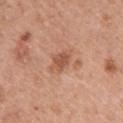| key | value |
|---|---|
| notes | catalogued during a skin exam; not biopsied |
| site | the left upper arm |
| imaging modality | total-body-photography crop, ~15 mm field of view |
| tile lighting | white-light illumination |
| subject | female, roughly 40 years of age |
| diameter | ~3 mm (longest diameter) |